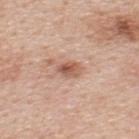Case summary:
– patient · male, about 50 years old
– lesion size · ~2.5 mm (longest diameter)
– image · ~15 mm tile from a whole-body skin photo
– site · the upper back
– lighting · white-light
– automated lesion analysis · a footprint of about 4 mm², an outline eccentricity of about 0.75 (0 = round, 1 = elongated), and two-axis asymmetry of about 0.25; an average lesion color of about L≈57 a*≈23 b*≈30 (CIELAB) and a lesion-to-skin contrast of about 8 (normalized; higher = more distinct)